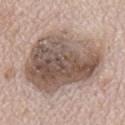This lesion was catalogued during total-body skin photography and was not selected for biopsy. Automated image analysis of the tile measured an area of roughly 55 mm², an outline eccentricity of about 0.55 (0 = round, 1 = elongated), and a symmetry-axis asymmetry near 0.25. It also reported an average lesion color of about L≈54 a*≈14 b*≈23 (CIELAB). On the mid back. A male subject, approximately 70 years of age. Measured at roughly 9.5 mm in maximum diameter. A 15 mm close-up tile from a total-body photography series done for melanoma screening. Captured under white-light illumination.